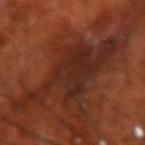notes: total-body-photography surveillance lesion; no biopsy | image: ~15 mm crop, total-body skin-cancer survey | location: the right forearm | patient: male, aged approximately 70 | diameter: ≈11 mm | illumination: cross-polarized.